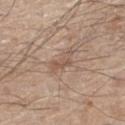Q: Was this lesion biopsied?
A: imaged on a skin check; not biopsied
Q: What lighting was used for the tile?
A: white-light illumination
Q: How was this image acquired?
A: ~15 mm tile from a whole-body skin photo
Q: Automated lesion metrics?
A: a lesion color around L≈54 a*≈17 b*≈27 in CIELAB, roughly 8 lightness units darker than nearby skin, and a normalized lesion–skin contrast near 5.5; border irregularity of about 6.5 on a 0–10 scale, a color-variation rating of about 2/10, and peripheral color asymmetry of about 0.5; a detector confidence of about 100 out of 100 that the crop contains a lesion
Q: Patient demographics?
A: male, in their 60s
Q: What is the anatomic site?
A: the leg